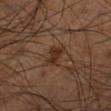Assessment:
This lesion was catalogued during total-body skin photography and was not selected for biopsy.
Image and clinical context:
The tile uses cross-polarized illumination. Longest diameter approximately 3 mm. An algorithmic analysis of the crop reported an outline eccentricity of about 0.7 (0 = round, 1 = elongated) and a shape-asymmetry score of about 0.3 (0 = symmetric). It also reported border irregularity of about 3 on a 0–10 scale, a within-lesion color-variation index near 2/10, and peripheral color asymmetry of about 0.5. And it measured a classifier nevus-likeness of about 45/100. A lesion tile, about 15 mm wide, cut from a 3D total-body photograph. A male patient, aged 58–62. The lesion is on the left lower leg.